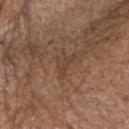Recorded during total-body skin imaging; not selected for excision or biopsy. Automated tile analysis of the lesion measured a lesion area of about 4 mm², a shape eccentricity near 0.8, and a symmetry-axis asymmetry near 0.65. It also reported an average lesion color of about L≈41 a*≈17 b*≈27 (CIELAB) and roughly 6 lightness units darker than nearby skin. A male subject, aged 78–82. A 15 mm close-up tile from a total-body photography series done for melanoma screening. On the head or neck. Imaged with white-light lighting.